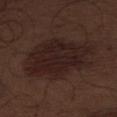Assessment:
The lesion was photographed on a routine skin check and not biopsied; there is no pathology result.
Clinical summary:
From the abdomen. This is a white-light tile. A male patient approximately 70 years of age. The recorded lesion diameter is about 9.5 mm. A 15 mm crop from a total-body photograph taken for skin-cancer surveillance.